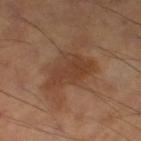Clinical impression: This lesion was catalogued during total-body skin photography and was not selected for biopsy. Clinical summary: A male subject aged 68–72. On the leg. A roughly 15 mm field-of-view crop from a total-body skin photograph. The lesion's longest dimension is about 6.5 mm.